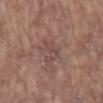Impression:
Recorded during total-body skin imaging; not selected for excision or biopsy.
Clinical summary:
This image is a 15 mm lesion crop taken from a total-body photograph. Imaged with white-light lighting. The total-body-photography lesion software estimated a lesion-to-skin contrast of about 4.5 (normalized; higher = more distinct). And it measured border irregularity of about 6 on a 0–10 scale, internal color variation of about 1.5 on a 0–10 scale, and radial color variation of about 0.5. It also reported lesion-presence confidence of about 70/100. A male subject roughly 65 years of age. About 3.5 mm across. From the mid back.